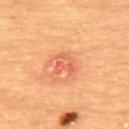Q: Is there a histopathology result?
A: no biopsy performed (imaged during a skin exam)
Q: How was the tile lit?
A: cross-polarized illumination
Q: Lesion location?
A: the upper back
Q: Lesion size?
A: ≈2.5 mm
Q: What kind of image is this?
A: ~15 mm crop, total-body skin-cancer survey
Q: Patient demographics?
A: female, in their 70s